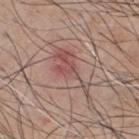Assessment: Imaged during a routine full-body skin examination; the lesion was not biopsied and no histopathology is available. Acquisition and patient details: A 15 mm close-up extracted from a 3D total-body photography capture. The patient is a male roughly 50 years of age. The lesion is located on the chest.